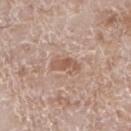The lesion was photographed on a routine skin check and not biopsied; there is no pathology result. About 3.5 mm across. A close-up tile cropped from a whole-body skin photograph, about 15 mm across. The subject is a male roughly 60 years of age. The lesion is located on the left lower leg. This is a white-light tile. The lesion-visualizer software estimated an average lesion color of about L≈56 a*≈19 b*≈28 (CIELAB), about 9 CIELAB-L* units darker than the surrounding skin, and a lesion-to-skin contrast of about 6.5 (normalized; higher = more distinct). The analysis additionally found an automated nevus-likeness rating near 0 out of 100 and lesion-presence confidence of about 100/100.